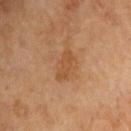tile lighting: cross-polarized illumination
image: 15 mm crop, total-body photography
lesion diameter: ≈4 mm
site: the left arm
patient: male, approximately 65 years of age